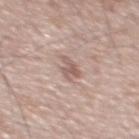Captured during whole-body skin photography for melanoma surveillance; the lesion was not biopsied.
A region of skin cropped from a whole-body photographic capture, roughly 15 mm wide.
The lesion is located on the chest.
Automated tile analysis of the lesion measured an automated nevus-likeness rating near 0 out of 100 and lesion-presence confidence of about 90/100.
The tile uses white-light illumination.
The recorded lesion diameter is about 3 mm.
A male subject, roughly 65 years of age.